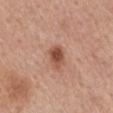workup: no biopsy performed (imaged during a skin exam) | size: ≈2.5 mm | anatomic site: the mid back | illumination: white-light | patient: male, in their mid- to late 50s | imaging modality: ~15 mm crop, total-body skin-cancer survey.